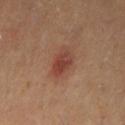Part of a total-body skin-imaging series; this lesion was reviewed on a skin check and was not flagged for biopsy. From the leg. A 15 mm crop from a total-body photograph taken for skin-cancer surveillance. The patient is a female aged 38–42. Captured under cross-polarized illumination.